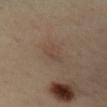<lesion>
  <biopsy_status>not biopsied; imaged during a skin examination</biopsy_status>
  <image>
    <source>total-body photography crop</source>
    <field_of_view_mm>15</field_of_view_mm>
  </image>
  <lesion_size>
    <long_diameter_mm_approx>4.0</long_diameter_mm_approx>
  </lesion_size>
  <patient>
    <sex>female</sex>
    <age_approx>40</age_approx>
  </patient>
  <lighting>cross-polarized</lighting>
  <site>upper back</site>
  <automated_metrics>
    <eccentricity>0.9</eccentricity>
    <nevus_likeness_0_100>5</nevus_likeness_0_100>
    <lesion_detection_confidence_0_100>100</lesion_detection_confidence_0_100>
  </automated_metrics>
</lesion>